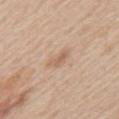{"biopsy_status": "not biopsied; imaged during a skin examination", "automated_metrics": {"area_mm2_approx": 3.0, "eccentricity": 0.85, "shape_asymmetry": 0.35, "border_irregularity_0_10": 3.5, "color_variation_0_10": 0.5, "nevus_likeness_0_100": 5, "lesion_detection_confidence_0_100": 100}, "lighting": "white-light", "site": "mid back", "patient": {"sex": "male", "age_approx": 60}, "image": {"source": "total-body photography crop", "field_of_view_mm": 15}, "lesion_size": {"long_diameter_mm_approx": 2.5}}